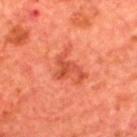This lesion was catalogued during total-body skin photography and was not selected for biopsy.
A 15 mm close-up tile from a total-body photography series done for melanoma screening.
Imaged with cross-polarized lighting.
Located on the back.
The patient is a male in their mid- to late 60s.